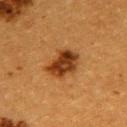<tbp_lesion>
  <biopsy_status>not biopsied; imaged during a skin examination</biopsy_status>
  <patient>
    <sex>female</sex>
    <age_approx>55</age_approx>
  </patient>
  <lighting>cross-polarized</lighting>
  <lesion_size>
    <long_diameter_mm_approx>4.0</long_diameter_mm_approx>
  </lesion_size>
  <image>
    <source>total-body photography crop</source>
    <field_of_view_mm>15</field_of_view_mm>
  </image>
  <automated_metrics>
    <area_mm2_approx>10.0</area_mm2_approx>
    <eccentricity>0.65</eccentricity>
    <shape_asymmetry>0.2</shape_asymmetry>
    <color_variation_0_10>5.5</color_variation_0_10>
    <peripheral_color_asymmetry>1.5</peripheral_color_asymmetry>
  </automated_metrics>
  <site>upper back</site>
</tbp_lesion>